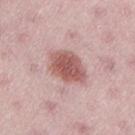Clinical impression: The lesion was photographed on a routine skin check and not biopsied; there is no pathology result. Acquisition and patient details: About 5 mm across. A female subject approximately 40 years of age. An algorithmic analysis of the crop reported a classifier nevus-likeness of about 90/100. On the lower back. A lesion tile, about 15 mm wide, cut from a 3D total-body photograph.